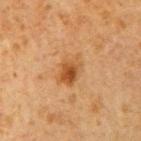Captured during whole-body skin photography for melanoma surveillance; the lesion was not biopsied.
The tile uses cross-polarized illumination.
Approximately 4 mm at its widest.
The patient is a male aged 58 to 62.
On the right upper arm.
A 15 mm crop from a total-body photograph taken for skin-cancer surveillance.